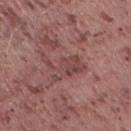Assessment: The lesion was tiled from a total-body skin photograph and was not biopsied. Acquisition and patient details: The patient is a female in their 20s. The lesion is on the right thigh. A lesion tile, about 15 mm wide, cut from a 3D total-body photograph.